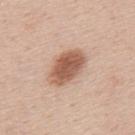Acquisition and patient details: Cropped from a total-body skin-imaging series; the visible field is about 15 mm. The lesion-visualizer software estimated a nevus-likeness score of about 95/100 and a detector confidence of about 100 out of 100 that the crop contains a lesion. Longest diameter approximately 5 mm. Located on the upper back. The tile uses white-light illumination. A male patient about 45 years old.